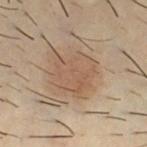biopsy status = imaged on a skin check; not biopsied
site = the chest
imaging modality = total-body-photography crop, ~15 mm field of view
patient = male, approximately 30 years of age